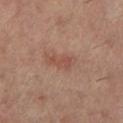This lesion was catalogued during total-body skin photography and was not selected for biopsy.
Imaged with cross-polarized lighting.
About 3.5 mm across.
The lesion is on the left lower leg.
The patient is a female approximately 60 years of age.
Automated tile analysis of the lesion measured a lesion area of about 5.5 mm², a shape eccentricity near 0.85, and a symmetry-axis asymmetry near 0.35. And it measured internal color variation of about 3 on a 0–10 scale and a peripheral color-asymmetry measure near 1.
This image is a 15 mm lesion crop taken from a total-body photograph.